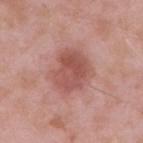{"biopsy_status": "not biopsied; imaged during a skin examination", "site": "arm", "image": {"source": "total-body photography crop", "field_of_view_mm": 15}, "lesion_size": {"long_diameter_mm_approx": 4.5}, "patient": {"sex": "male", "age_approx": 55}, "automated_metrics": {"border_irregularity_0_10": 2.5, "color_variation_0_10": 4.0, "peripheral_color_asymmetry": 1.5}}